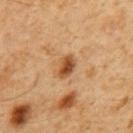Part of a total-body skin-imaging series; this lesion was reviewed on a skin check and was not flagged for biopsy.